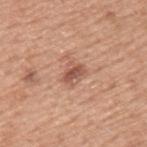The lesion was tiled from a total-body skin photograph and was not biopsied.
Automated tile analysis of the lesion measured an average lesion color of about L≈54 a*≈23 b*≈29 (CIELAB), roughly 11 lightness units darker than nearby skin, and a normalized lesion–skin contrast near 7.5. It also reported border irregularity of about 2 on a 0–10 scale, a color-variation rating of about 4.5/10, and a peripheral color-asymmetry measure near 1.5. It also reported a classifier nevus-likeness of about 40/100 and a lesion-detection confidence of about 100/100.
On the left upper arm.
Cropped from a whole-body photographic skin survey; the tile spans about 15 mm.
Measured at roughly 2.5 mm in maximum diameter.
The subject is a male roughly 50 years of age.